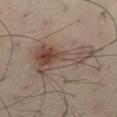  biopsy_status: not biopsied; imaged during a skin examination
  automated_metrics:
    area_mm2_approx: 22.0
    eccentricity: 0.9
    shape_asymmetry: 0.5
    vs_skin_contrast_norm: 7.0
    color_variation_0_10: 9.0
    peripheral_color_asymmetry: 3.0
    nevus_likeness_0_100: 85
    lesion_detection_confidence_0_100: 100
  site: abdomen
  lighting: cross-polarized
  lesion_size:
    long_diameter_mm_approx: 8.5
  patient:
    sex: male
    age_approx: 55
  image:
    source: total-body photography crop
    field_of_view_mm: 15